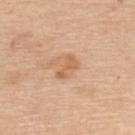Assessment: No biopsy was performed on this lesion — it was imaged during a full skin examination and was not determined to be concerning. Acquisition and patient details: The lesion's longest dimension is about 3 mm. Located on the upper back. A female subject, in their mid-60s. Automated image analysis of the tile measured a nevus-likeness score of about 0/100 and lesion-presence confidence of about 100/100. A 15 mm close-up tile from a total-body photography series done for melanoma screening. Captured under white-light illumination.